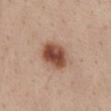Imaged during a routine full-body skin examination; the lesion was not biopsied and no histopathology is available. An algorithmic analysis of the crop reported a footprint of about 11 mm², an eccentricity of roughly 0.75, and a symmetry-axis asymmetry near 0.15. The software also gave a lesion color around L≈50 a*≈22 b*≈30 in CIELAB, about 16 CIELAB-L* units darker than the surrounding skin, and a lesion-to-skin contrast of about 11 (normalized; higher = more distinct). And it measured border irregularity of about 1.5 on a 0–10 scale, a within-lesion color-variation index near 7/10, and a peripheral color-asymmetry measure near 2. The subject is a male aged 53–57. Captured under white-light illumination. The lesion is on the abdomen. Cropped from a whole-body photographic skin survey; the tile spans about 15 mm.